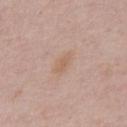Assessment:
The lesion was tiled from a total-body skin photograph and was not biopsied.
Context:
The lesion is located on the chest. Longest diameter approximately 3.5 mm. The patient is a male aged around 50. This image is a 15 mm lesion crop taken from a total-body photograph.